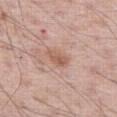Case summary:
– notes: total-body-photography surveillance lesion; no biopsy
– acquisition: ~15 mm tile from a whole-body skin photo
– lesion size: ~3 mm (longest diameter)
– patient: male, approximately 60 years of age
– anatomic site: the right thigh